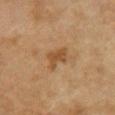Impression:
No biopsy was performed on this lesion — it was imaged during a full skin examination and was not determined to be concerning.
Clinical summary:
The patient is a female aged 58–62. This is a cross-polarized tile. From the chest. The lesion-visualizer software estimated roughly 8 lightness units darker than nearby skin and a lesion-to-skin contrast of about 7 (normalized; higher = more distinct). The analysis additionally found a border-irregularity index near 4.5/10, internal color variation of about 1.5 on a 0–10 scale, and radial color variation of about 0.5. This image is a 15 mm lesion crop taken from a total-body photograph. About 3 mm across.